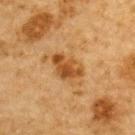The lesion was tiled from a total-body skin photograph and was not biopsied.
The lesion is located on the upper back.
A male subject in their mid-80s or older.
Cropped from a whole-body photographic skin survey; the tile spans about 15 mm.